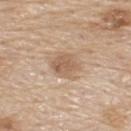Recorded during total-body skin imaging; not selected for excision or biopsy. A male patient, aged 78 to 82. Imaged with white-light lighting. Located on the upper back. A 15 mm close-up tile from a total-body photography series done for melanoma screening.